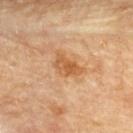<tbp_lesion>
  <biopsy_status>not biopsied; imaged during a skin examination</biopsy_status>
  <lesion_size>
    <long_diameter_mm_approx>3.5</long_diameter_mm_approx>
  </lesion_size>
  <lighting>cross-polarized</lighting>
  <image>
    <source>total-body photography crop</source>
    <field_of_view_mm>15</field_of_view_mm>
  </image>
  <site>upper back</site>
  <patient>
    <sex>male</sex>
    <age_approx>85+</age_approx>
  </patient>
</tbp_lesion>